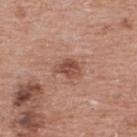tile lighting=white-light illumination; acquisition=total-body-photography crop, ~15 mm field of view; patient=male, aged approximately 70; anatomic site=the back.